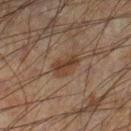Assessment:
The lesion was tiled from a total-body skin photograph and was not biopsied.
Background:
The lesion's longest dimension is about 3 mm. The tile uses cross-polarized illumination. The lesion-visualizer software estimated a footprint of about 5 mm², an outline eccentricity of about 0.75 (0 = round, 1 = elongated), and a shape-asymmetry score of about 0.35 (0 = symmetric). And it measured a mean CIELAB color near L≈28 a*≈14 b*≈22, a lesion–skin lightness drop of about 7, and a normalized border contrast of about 8. The analysis additionally found a border-irregularity rating of about 3.5/10, a within-lesion color-variation index near 3/10, and peripheral color asymmetry of about 1.5. A 15 mm close-up extracted from a 3D total-body photography capture. Located on the leg. The patient is a male aged around 60.